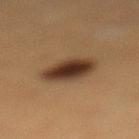Captured during whole-body skin photography for melanoma surveillance; the lesion was not biopsied.
The subject is a female approximately 40 years of age.
Cropped from a total-body skin-imaging series; the visible field is about 15 mm.
About 4.5 mm across.
From the left lower leg.
Captured under cross-polarized illumination.